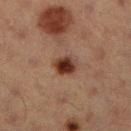The lesion was tiled from a total-body skin photograph and was not biopsied.